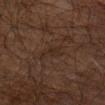Q: Patient demographics?
A: male, aged 58–62
Q: Illumination type?
A: cross-polarized
Q: Lesion location?
A: the mid back
Q: How was this image acquired?
A: 15 mm crop, total-body photography